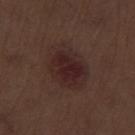No biopsy was performed on this lesion — it was imaged during a full skin examination and was not determined to be concerning.
Longest diameter approximately 6 mm.
A lesion tile, about 15 mm wide, cut from a 3D total-body photograph.
A male patient, aged 68–72.
The lesion is on the leg.